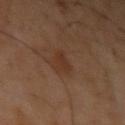Case summary:
• workup: catalogued during a skin exam; not biopsied
• location: the left upper arm
• patient: male, aged 58–62
• illumination: cross-polarized illumination
• image source: ~15 mm crop, total-body skin-cancer survey